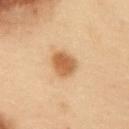Clinical impression:
The lesion was tiled from a total-body skin photograph and was not biopsied.
Acquisition and patient details:
From the front of the torso. The tile uses cross-polarized illumination. A male patient aged 53 to 57. Measured at roughly 3.5 mm in maximum diameter. Cropped from a whole-body photographic skin survey; the tile spans about 15 mm.